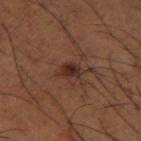The lesion was tiled from a total-body skin photograph and was not biopsied. This is a cross-polarized tile. A male subject, about 65 years old. This image is a 15 mm lesion crop taken from a total-body photograph. The total-body-photography lesion software estimated a lesion color around L≈29 a*≈20 b*≈24 in CIELAB, a lesion–skin lightness drop of about 9, and a normalized border contrast of about 9.5. The analysis additionally found a border-irregularity index near 2.5/10, a color-variation rating of about 3.5/10, and a peripheral color-asymmetry measure near 1. On the left thigh. The recorded lesion diameter is about 2.5 mm.